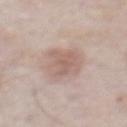biopsy status: imaged on a skin check; not biopsied | size: about 5 mm | imaging modality: total-body-photography crop, ~15 mm field of view | location: the abdomen | subject: male, in their mid-70s.